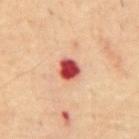Recorded during total-body skin imaging; not selected for excision or biopsy. This is a cross-polarized tile. A male patient, about 70 years old. Located on the back. Cropped from a whole-body photographic skin survey; the tile spans about 15 mm.